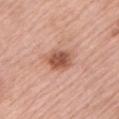notes: imaged on a skin check; not biopsied | anatomic site: the left upper arm | lesion size: ~3.5 mm (longest diameter) | TBP lesion metrics: border irregularity of about 1.5 on a 0–10 scale, a within-lesion color-variation index near 4/10, and peripheral color asymmetry of about 1; a detector confidence of about 100 out of 100 that the crop contains a lesion | patient: male, in their mid-70s | tile lighting: white-light | imaging modality: ~15 mm crop, total-body skin-cancer survey.